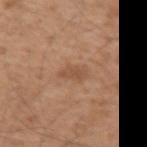follow-up — no biopsy performed (imaged during a skin exam)
image — ~15 mm tile from a whole-body skin photo
lesion size — about 2.5 mm
site — the left upper arm
lighting — white-light illumination
automated lesion analysis — a footprint of about 3 mm² and an eccentricity of roughly 0.8; a lesion color around L≈51 a*≈21 b*≈32 in CIELAB and a lesion–skin lightness drop of about 8
patient — male, aged 48–52